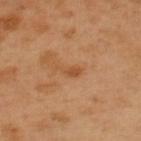<lesion>
<patient>
  <sex>male</sex>
  <age_approx>50</age_approx>
</patient>
<lighting>cross-polarized</lighting>
<site>upper back</site>
<lesion_size>
  <long_diameter_mm_approx>3.0</long_diameter_mm_approx>
</lesion_size>
<image>
  <source>total-body photography crop</source>
  <field_of_view_mm>15</field_of_view_mm>
</image>
</lesion>